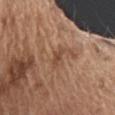subject: male, aged 73–77; size: ≈2.5 mm; anatomic site: the chest; image source: ~15 mm tile from a whole-body skin photo.